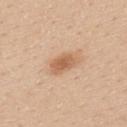| key | value |
|---|---|
| biopsy status | catalogued during a skin exam; not biopsied |
| lesion size | about 3.5 mm |
| image | ~15 mm tile from a whole-body skin photo |
| lighting | white-light illumination |
| site | the back |
| patient | male, roughly 30 years of age |